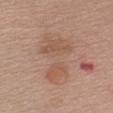biopsy status — total-body-photography surveillance lesion; no biopsy
diameter — ≈6.5 mm
image source — ~15 mm crop, total-body skin-cancer survey
anatomic site — the chest
TBP lesion metrics — an outline eccentricity of about 0.75 (0 = round, 1 = elongated); a nevus-likeness score of about 0/100 and lesion-presence confidence of about 100/100
subject — female, aged 63–67